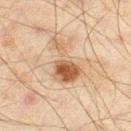follow-up = no biopsy performed (imaged during a skin exam)
tile lighting = cross-polarized illumination
site = the right thigh
size = ≈6 mm
acquisition = ~15 mm tile from a whole-body skin photo
subject = male, in their mid- to late 40s